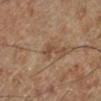Q: What is the lesion's diameter?
A: ≈2.5 mm
Q: What are the patient's age and sex?
A: male, roughly 70 years of age
Q: How was the tile lit?
A: cross-polarized illumination
Q: How was this image acquired?
A: ~15 mm crop, total-body skin-cancer survey
Q: What is the anatomic site?
A: the left lower leg